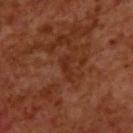| feature | finding |
|---|---|
| notes | catalogued during a skin exam; not biopsied |
| diameter | ≈3 mm |
| subject | male, roughly 70 years of age |
| illumination | cross-polarized |
| imaging modality | ~15 mm tile from a whole-body skin photo |
| image-analysis metrics | an outline eccentricity of about 0.9 (0 = round, 1 = elongated) and two-axis asymmetry of about 0.4; a lesion color around L≈28 a*≈25 b*≈30 in CIELAB, a lesion–skin lightness drop of about 5, and a lesion-to-skin contrast of about 5 (normalized; higher = more distinct); a detector confidence of about 95 out of 100 that the crop contains a lesion |
| anatomic site | the upper back |